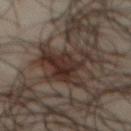On the front of the torso. This image is a 15 mm lesion crop taken from a total-body photograph. The patient is a male roughly 65 years of age.